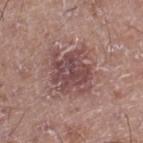Assessment: The lesion was tiled from a total-body skin photograph and was not biopsied. Image and clinical context: The patient is a male aged around 20. Located on the right lower leg. Automated tile analysis of the lesion measured a lesion area of about 18 mm² and an eccentricity of roughly 0.45. And it measured a lesion color around L≈46 a*≈21 b*≈19 in CIELAB and about 10 CIELAB-L* units darker than the surrounding skin. And it measured border irregularity of about 5.5 on a 0–10 scale. The software also gave a classifier nevus-likeness of about 0/100 and a lesion-detection confidence of about 100/100. About 5 mm across. The tile uses white-light illumination. A close-up tile cropped from a whole-body skin photograph, about 15 mm across.